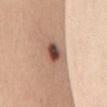Assessment:
Imaged during a routine full-body skin examination; the lesion was not biopsied and no histopathology is available.
Context:
The recorded lesion diameter is about 4.5 mm. The lesion-visualizer software estimated an outline eccentricity of about 0.8 (0 = round, 1 = elongated) and a shape-asymmetry score of about 0.2 (0 = symmetric). The analysis additionally found border irregularity of about 2 on a 0–10 scale, a within-lesion color-variation index near 9/10, and peripheral color asymmetry of about 2.5. A 15 mm close-up extracted from a 3D total-body photography capture. The subject is a female roughly 30 years of age. From the front of the torso.